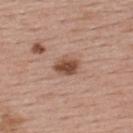Captured during whole-body skin photography for melanoma surveillance; the lesion was not biopsied.
Located on the upper back.
This image is a 15 mm lesion crop taken from a total-body photograph.
A female patient aged 53–57.